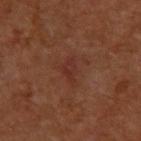This lesion was catalogued during total-body skin photography and was not selected for biopsy.
The subject is a male about 60 years old.
The lesion is located on the upper back.
The tile uses cross-polarized illumination.
The lesion's longest dimension is about 3.5 mm.
A lesion tile, about 15 mm wide, cut from a 3D total-body photograph.